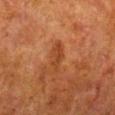biopsy status: total-body-photography surveillance lesion; no biopsy | tile lighting: cross-polarized illumination | automated metrics: a footprint of about 4 mm², an eccentricity of roughly 0.9, and a symmetry-axis asymmetry near 0.45; a lesion–skin lightness drop of about 6; a within-lesion color-variation index near 1.5/10 | subject: male, aged 78–82 | size: ~3.5 mm (longest diameter) | image source: ~15 mm tile from a whole-body skin photo | body site: the leg.